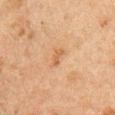follow-up — no biopsy performed (imaged during a skin exam)
anatomic site — the right upper arm
image-analysis metrics — a footprint of about 3 mm², a shape eccentricity near 0.9, and two-axis asymmetry of about 0.3; border irregularity of about 3 on a 0–10 scale and internal color variation of about 0 on a 0–10 scale; a lesion-detection confidence of about 100/100
illumination — cross-polarized
image source — ~15 mm tile from a whole-body skin photo
patient — male, in their 50s
diameter — ≈2.5 mm